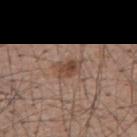Q: Was a biopsy performed?
A: catalogued during a skin exam; not biopsied
Q: What did automated image analysis measure?
A: an area of roughly 4.5 mm², an outline eccentricity of about 0.7 (0 = round, 1 = elongated), and a symmetry-axis asymmetry near 0.25; an average lesion color of about L≈45 a*≈19 b*≈27 (CIELAB), roughly 10 lightness units darker than nearby skin, and a lesion-to-skin contrast of about 8 (normalized; higher = more distinct); an automated nevus-likeness rating near 80 out of 100 and a lesion-detection confidence of about 100/100
Q: What kind of image is this?
A: total-body-photography crop, ~15 mm field of view
Q: How large is the lesion?
A: about 3 mm
Q: How was the tile lit?
A: white-light
Q: What is the anatomic site?
A: the mid back
Q: Patient demographics?
A: male, in their 60s